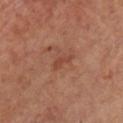Q: Was a biopsy performed?
A: no biopsy performed (imaged during a skin exam)
Q: How large is the lesion?
A: ≈2.5 mm
Q: What did automated image analysis measure?
A: a border-irregularity rating of about 3.5/10 and a within-lesion color-variation index near 0/10; an automated nevus-likeness rating near 0 out of 100 and a detector confidence of about 100 out of 100 that the crop contains a lesion
Q: Patient demographics?
A: female, about 45 years old
Q: Lesion location?
A: the chest
Q: How was this image acquired?
A: ~15 mm crop, total-body skin-cancer survey